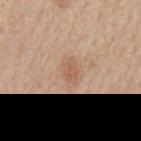Findings:
– notes · total-body-photography surveillance lesion; no biopsy
– subject · male, roughly 60 years of age
– location · the back
– lesion diameter · about 3 mm
– lighting · white-light illumination
– imaging modality · ~15 mm tile from a whole-body skin photo